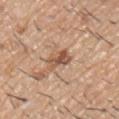Q: Was this lesion biopsied?
A: no biopsy performed (imaged during a skin exam)
Q: What is the imaging modality?
A: ~15 mm crop, total-body skin-cancer survey
Q: Lesion size?
A: ~3.5 mm (longest diameter)
Q: Illumination type?
A: white-light illumination
Q: Where on the body is the lesion?
A: the left upper arm
Q: Who is the patient?
A: male, roughly 60 years of age